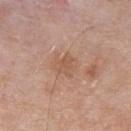Impression:
Part of a total-body skin-imaging series; this lesion was reviewed on a skin check and was not flagged for biopsy.
Acquisition and patient details:
Located on the chest. The subject is a male about 55 years old. This is a white-light tile. A 15 mm crop from a total-body photograph taken for skin-cancer surveillance.